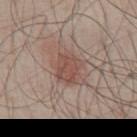Findings:
– workup — no biopsy performed (imaged during a skin exam)
– patient — male, about 50 years old
– lesion diameter — ~3.5 mm (longest diameter)
– imaging modality — total-body-photography crop, ~15 mm field of view
– tile lighting — white-light illumination
– image-analysis metrics — an area of roughly 7.5 mm², an eccentricity of roughly 0.65, and a shape-asymmetry score of about 0.2 (0 = symmetric); a mean CIELAB color near L≈49 a*≈20 b*≈24, roughly 8 lightness units darker than nearby skin, and a lesion-to-skin contrast of about 6.5 (normalized; higher = more distinct)
– location — the chest